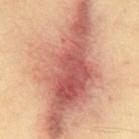biopsy status — no biopsy performed (imaged during a skin exam)
patient — male, aged 38–42
lighting — cross-polarized illumination
image — ~15 mm crop, total-body skin-cancer survey
automated lesion analysis — a lesion area of about 90 mm², an outline eccentricity of about 0.85 (0 = round, 1 = elongated), and a symmetry-axis asymmetry near 0.25; a border-irregularity rating of about 4.5/10, a color-variation rating of about 10/10, and peripheral color asymmetry of about 3.5; a classifier nevus-likeness of about 15/100 and lesion-presence confidence of about 90/100
lesion diameter — about 16.5 mm
anatomic site — the front of the torso